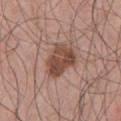Captured during whole-body skin photography for melanoma surveillance; the lesion was not biopsied. A male patient, approximately 40 years of age. Cropped from a whole-body photographic skin survey; the tile spans about 15 mm. Located on the chest.